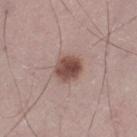Clinical impression: Captured during whole-body skin photography for melanoma surveillance; the lesion was not biopsied. Context: From the left thigh. The patient is a male in their mid-40s. A close-up tile cropped from a whole-body skin photograph, about 15 mm across. Captured under white-light illumination. An algorithmic analysis of the crop reported a border-irregularity index near 1/10 and a within-lesion color-variation index near 4.5/10. And it measured an automated nevus-likeness rating near 95 out of 100 and a lesion-detection confidence of about 100/100.